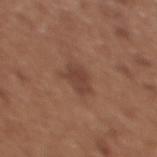  biopsy_status: not biopsied; imaged during a skin examination
  site: chest
  image:
    source: total-body photography crop
    field_of_view_mm: 15
  patient:
    sex: female
    age_approx: 35